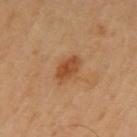The lesion was photographed on a routine skin check and not biopsied; there is no pathology result. From the left upper arm. A male subject in their mid-50s. This image is a 15 mm lesion crop taken from a total-body photograph.